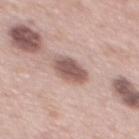Q: Was this lesion biopsied?
A: total-body-photography surveillance lesion; no biopsy
Q: What kind of image is this?
A: total-body-photography crop, ~15 mm field of view
Q: What is the lesion's diameter?
A: ≈4 mm
Q: Who is the patient?
A: male, roughly 65 years of age
Q: What is the anatomic site?
A: the back
Q: What lighting was used for the tile?
A: white-light